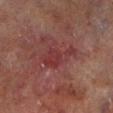<tbp_lesion>
  <biopsy_status>not biopsied; imaged during a skin examination</biopsy_status>
  <site>leg</site>
  <automated_metrics>
    <border_irregularity_0_10>5.0</border_irregularity_0_10>
    <color_variation_0_10>2.5</color_variation_0_10>
    <peripheral_color_asymmetry>1.0</peripheral_color_asymmetry>
    <nevus_likeness_0_100>0</nevus_likeness_0_100>
  </automated_metrics>
  <lesion_size>
    <long_diameter_mm_approx>5.0</long_diameter_mm_approx>
  </lesion_size>
  <patient>
    <sex>male</sex>
    <age_approx>70</age_approx>
  </patient>
  <image>
    <source>total-body photography crop</source>
    <field_of_view_mm>15</field_of_view_mm>
  </image>
</tbp_lesion>